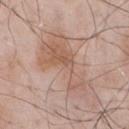| feature | finding |
|---|---|
| follow-up | no biopsy performed (imaged during a skin exam) |
| subject | male, approximately 55 years of age |
| lesion size | about 9 mm |
| acquisition | ~15 mm crop, total-body skin-cancer survey |
| body site | the front of the torso |
| tile lighting | white-light |
| image-analysis metrics | a lesion color around L≈59 a*≈18 b*≈28 in CIELAB and roughly 8 lightness units darker than nearby skin |